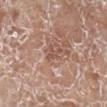A female subject, aged approximately 75.
Imaged with white-light lighting.
The lesion-visualizer software estimated an eccentricity of roughly 0.9 and a shape-asymmetry score of about 0.35 (0 = symmetric). The software also gave a mean CIELAB color near L≈52 a*≈20 b*≈28, about 8 CIELAB-L* units darker than the surrounding skin, and a normalized border contrast of about 6.
A close-up tile cropped from a whole-body skin photograph, about 15 mm across.
Approximately 2.5 mm at its widest.
On the left lower leg.